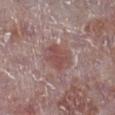Q: Is there a histopathology result?
A: imaged on a skin check; not biopsied
Q: What is the imaging modality?
A: ~15 mm crop, total-body skin-cancer survey
Q: Illumination type?
A: white-light illumination
Q: What is the anatomic site?
A: the right lower leg
Q: Who is the patient?
A: male, in their mid- to late 70s
Q: How large is the lesion?
A: about 4 mm